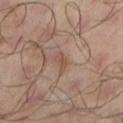notes = catalogued during a skin exam; not biopsied | automated lesion analysis = a within-lesion color-variation index near 0/10 and radial color variation of about 0; a nevus-likeness score of about 0/100 | anatomic site = the left lower leg | image source = ~15 mm tile from a whole-body skin photo | patient = male, roughly 65 years of age.